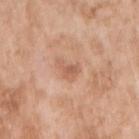Image and clinical context: Located on the right upper arm. The total-body-photography lesion software estimated a border-irregularity rating of about 5.5/10, internal color variation of about 2 on a 0–10 scale, and a peripheral color-asymmetry measure near 1. A 15 mm close-up tile from a total-body photography series done for melanoma screening. A female patient, approximately 75 years of age.